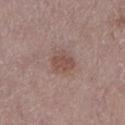A 15 mm crop from a total-body photograph taken for skin-cancer surveillance.
A female patient, aged 63 to 67.
The lesion's longest dimension is about 3 mm.
Located on the right lower leg.
Captured under white-light illumination.